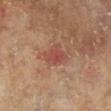Acquisition and patient details: Located on the right lower leg. The patient is a male aged around 65. Approximately 3 mm at its widest. A 15 mm close-up extracted from a 3D total-body photography capture. Imaged with cross-polarized lighting. The lesion-visualizer software estimated an eccentricity of roughly 0.65 and two-axis asymmetry of about 0.3.